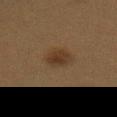biopsy_status: not biopsied; imaged during a skin examination
image:
  source: total-body photography crop
  field_of_view_mm: 15
patient:
  sex: female
  age_approx: 40
lesion_size:
  long_diameter_mm_approx: 3.0
lighting: cross-polarized
automated_metrics:
  area_mm2_approx: 6.0
  shape_asymmetry: 0.2
  cielab_L: 27
  cielab_a: 13
  cielab_b: 24
  vs_skin_darker_L: 6.0
site: abdomen